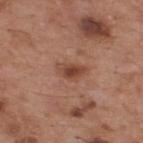No biopsy was performed on this lesion — it was imaged during a full skin examination and was not determined to be concerning. About 3.5 mm across. The lesion-visualizer software estimated a footprint of about 5.5 mm² and an outline eccentricity of about 0.8 (0 = round, 1 = elongated). And it measured an average lesion color of about L≈45 a*≈23 b*≈29 (CIELAB) and about 10 CIELAB-L* units darker than the surrounding skin. The analysis additionally found a border-irregularity index near 3/10, internal color variation of about 3.5 on a 0–10 scale, and radial color variation of about 1. Captured under white-light illumination. A male subject, roughly 55 years of age. A region of skin cropped from a whole-body photographic capture, roughly 15 mm wide. The lesion is on the upper back.